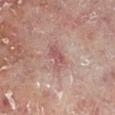• subject: male, approximately 60 years of age
• tile lighting: cross-polarized
• acquisition: total-body-photography crop, ~15 mm field of view
• anatomic site: the left lower leg
• size: ≈3 mm
• image-analysis metrics: a border-irregularity rating of about 3/10, internal color variation of about 2 on a 0–10 scale, and a peripheral color-asymmetry measure near 0.5; a nevus-likeness score of about 0/100 and lesion-presence confidence of about 100/100